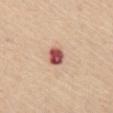Assessment:
Part of a total-body skin-imaging series; this lesion was reviewed on a skin check and was not flagged for biopsy.
Acquisition and patient details:
A female patient in their mid-60s. Captured under white-light illumination. A lesion tile, about 15 mm wide, cut from a 3D total-body photograph. From the mid back.